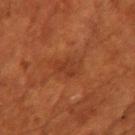* notes: imaged on a skin check; not biopsied
* lighting: cross-polarized illumination
* lesion diameter: ≈3 mm
* acquisition: 15 mm crop, total-body photography
* body site: the upper back
* image-analysis metrics: a mean CIELAB color near L≈28 a*≈21 b*≈27, about 5 CIELAB-L* units darker than the surrounding skin, and a normalized lesion–skin contrast near 5.5; border irregularity of about 3 on a 0–10 scale
* patient: female, aged approximately 80